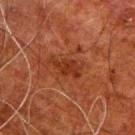<lesion>
  <biopsy_status>not biopsied; imaged during a skin examination</biopsy_status>
  <lesion_size>
    <long_diameter_mm_approx>4.0</long_diameter_mm_approx>
  </lesion_size>
  <patient>
    <sex>male</sex>
    <age_approx>80</age_approx>
  </patient>
  <site>right upper arm</site>
  <image>
    <source>total-body photography crop</source>
    <field_of_view_mm>15</field_of_view_mm>
  </image>
  <lighting>cross-polarized</lighting>
</lesion>